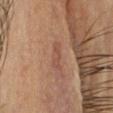Clinical impression:
The lesion was tiled from a total-body skin photograph and was not biopsied.
Context:
Imaged with cross-polarized lighting. A male patient approximately 45 years of age. The total-body-photography lesion software estimated lesion-presence confidence of about 100/100. About 3 mm across. This image is a 15 mm lesion crop taken from a total-body photograph. The lesion is on the head or neck.